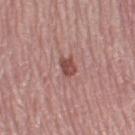biopsy status = total-body-photography surveillance lesion; no biopsy.